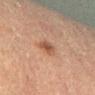notes=no biopsy performed (imaged during a skin exam); patient=male, aged 83 or over; body site=the left lower leg; image=15 mm crop, total-body photography; illumination=cross-polarized illumination.